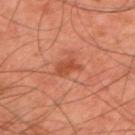– follow-up: imaged on a skin check; not biopsied
– anatomic site: the upper back
– imaging modality: ~15 mm crop, total-body skin-cancer survey
– diameter: ≈3 mm
– subject: male, aged 43 to 47
– tile lighting: cross-polarized illumination